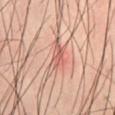<tbp_lesion>
  <biopsy_status>not biopsied; imaged during a skin examination</biopsy_status>
  <patient>
    <sex>male</sex>
    <age_approx>40</age_approx>
  </patient>
  <lesion_size>
    <long_diameter_mm_approx>4.5</long_diameter_mm_approx>
  </lesion_size>
  <image>
    <source>total-body photography crop</source>
    <field_of_view_mm>15</field_of_view_mm>
  </image>
  <site>back</site>
</tbp_lesion>